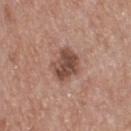Q: Is there a histopathology result?
A: no biopsy performed (imaged during a skin exam)
Q: What kind of image is this?
A: 15 mm crop, total-body photography
Q: What is the lesion's diameter?
A: about 3.5 mm
Q: Automated lesion metrics?
A: a lesion area of about 10 mm² and a shape eccentricity near 0.45; a border-irregularity rating of about 2/10 and a within-lesion color-variation index near 4.5/10
Q: What are the patient's age and sex?
A: male, approximately 55 years of age
Q: How was the tile lit?
A: white-light